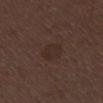Clinical impression: The lesion was photographed on a routine skin check and not biopsied; there is no pathology result. Acquisition and patient details: The lesion is located on the right thigh. Approximately 3 mm at its widest. This is a white-light tile. A female subject, aged 48 to 52. Cropped from a total-body skin-imaging series; the visible field is about 15 mm.